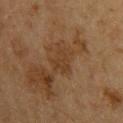Part of a total-body skin-imaging series; this lesion was reviewed on a skin check and was not flagged for biopsy.
The tile uses cross-polarized illumination.
The lesion-visualizer software estimated an area of roughly 7 mm², an outline eccentricity of about 0.65 (0 = round, 1 = elongated), and a symmetry-axis asymmetry near 0.4.
The recorded lesion diameter is about 3.5 mm.
A region of skin cropped from a whole-body photographic capture, roughly 15 mm wide.
A male subject aged 58–62.